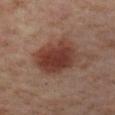Case summary:
- workup: total-body-photography surveillance lesion; no biopsy
- location: the left lower leg
- tile lighting: cross-polarized
- image: 15 mm crop, total-body photography
- patient: female, aged 53 to 57
- lesion size: ≈5.5 mm
- TBP lesion metrics: an area of roughly 20 mm², an outline eccentricity of about 0.5 (0 = round, 1 = elongated), and a symmetry-axis asymmetry near 0.15; a classifier nevus-likeness of about 100/100 and a lesion-detection confidence of about 100/100